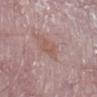Clinical impression: This lesion was catalogued during total-body skin photography and was not selected for biopsy. Image and clinical context: Imaged with white-light lighting. A female subject, aged approximately 65. The lesion-visualizer software estimated a lesion area of about 3.5 mm², an outline eccentricity of about 0.5 (0 = round, 1 = elongated), and a shape-asymmetry score of about 0.3 (0 = symmetric). And it measured a mean CIELAB color near L≈54 a*≈21 b*≈23 and a normalized border contrast of about 5. And it measured border irregularity of about 3.5 on a 0–10 scale and internal color variation of about 1 on a 0–10 scale. The recorded lesion diameter is about 2.5 mm. A roughly 15 mm field-of-view crop from a total-body skin photograph. The lesion is on the right lower leg.